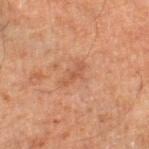{
  "image": {
    "source": "total-body photography crop",
    "field_of_view_mm": 15
  },
  "patient": {
    "sex": "male",
    "age_approx": 65
  },
  "site": "right lower leg"
}